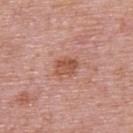Findings:
- follow-up: total-body-photography surveillance lesion; no biopsy
- size: ≈3 mm
- anatomic site: the upper back
- illumination: white-light illumination
- image source: 15 mm crop, total-body photography
- patient: male, in their mid-70s